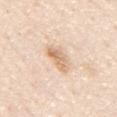| key | value |
|---|---|
| follow-up | imaged on a skin check; not biopsied |
| imaging modality | ~15 mm crop, total-body skin-cancer survey |
| patient | male, aged 78 to 82 |
| size | about 4 mm |
| location | the mid back |